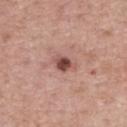Impression: The lesion was tiled from a total-body skin photograph and was not biopsied. Background: A male patient about 65 years old. A 15 mm close-up extracted from a 3D total-body photography capture. From the mid back.